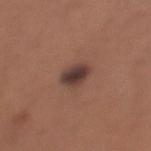Part of a total-body skin-imaging series; this lesion was reviewed on a skin check and was not flagged for biopsy.
About 3.5 mm across.
A close-up tile cropped from a whole-body skin photograph, about 15 mm across.
The lesion is on the left lower leg.
This is a white-light tile.
A female patient, about 30 years old.